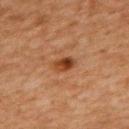{
  "biopsy_status": "not biopsied; imaged during a skin examination",
  "image": {
    "source": "total-body photography crop",
    "field_of_view_mm": 15
  },
  "patient": {
    "sex": "female",
    "age_approx": 60
  },
  "site": "upper back"
}